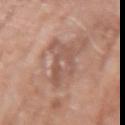Captured during whole-body skin photography for melanoma surveillance; the lesion was not biopsied. On the arm. Cropped from a total-body skin-imaging series; the visible field is about 15 mm. The total-body-photography lesion software estimated a lesion area of about 13 mm², an eccentricity of roughly 0.8, and a symmetry-axis asymmetry near 0.45. It also reported a nevus-likeness score of about 0/100 and a lesion-detection confidence of about 95/100. A female subject in their mid-70s.